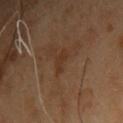Assessment: Recorded during total-body skin imaging; not selected for excision or biopsy. Clinical summary: Captured under cross-polarized illumination. Located on the front of the torso. The lesion's longest dimension is about 2.5 mm. Automated image analysis of the tile measured an eccentricity of roughly 0.85 and a symmetry-axis asymmetry near 0.5. The software also gave a border-irregularity rating of about 5.5/10. A lesion tile, about 15 mm wide, cut from a 3D total-body photograph. A male patient, aged approximately 55.